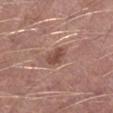  patient:
    sex: male
    age_approx: 55
  lighting: white-light
  lesion_size:
    long_diameter_mm_approx: 2.5
  image:
    source: total-body photography crop
    field_of_view_mm: 15
  automated_metrics:
    area_mm2_approx: 4.5
    eccentricity: 0.65
    shape_asymmetry: 0.25
  site: right lower leg